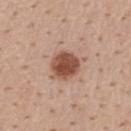  lesion_size:
    long_diameter_mm_approx: 3.5
  site: mid back
  patient:
    sex: male
    age_approx: 55
  image:
    source: total-body photography crop
    field_of_view_mm: 15